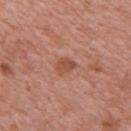Clinical impression:
Imaged during a routine full-body skin examination; the lesion was not biopsied and no histopathology is available.
Context:
The recorded lesion diameter is about 2.5 mm. A female subject aged approximately 40. The lesion is located on the upper back. Imaged with white-light lighting. The lesion-visualizer software estimated an average lesion color of about L≈51 a*≈25 b*≈31 (CIELAB) and a lesion-to-skin contrast of about 6.5 (normalized; higher = more distinct). A 15 mm close-up extracted from a 3D total-body photography capture.